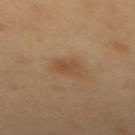| field | value |
|---|---|
| workup | imaged on a skin check; not biopsied |
| lesion diameter | about 3 mm |
| subject | female, aged approximately 55 |
| illumination | cross-polarized illumination |
| automated metrics | an average lesion color of about L≈48 a*≈17 b*≈35 (CIELAB) and roughly 7 lightness units darker than nearby skin; a color-variation rating of about 2/10 and radial color variation of about 1 |
| body site | the mid back |
| image | ~15 mm tile from a whole-body skin photo |